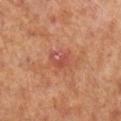No biopsy was performed on this lesion — it was imaged during a full skin examination and was not determined to be concerning.
A 15 mm close-up extracted from a 3D total-body photography capture.
From the right lower leg.
The subject is a female in their mid-60s.
This is a cross-polarized tile.
Automated tile analysis of the lesion measured border irregularity of about 2 on a 0–10 scale, a within-lesion color-variation index near 6/10, and peripheral color asymmetry of about 2. And it measured an automated nevus-likeness rating near 0 out of 100 and a lesion-detection confidence of about 100/100.
Measured at roughly 2.5 mm in maximum diameter.